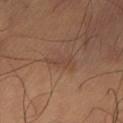Imaged during a routine full-body skin examination; the lesion was not biopsied and no histopathology is available. The total-body-photography lesion software estimated border irregularity of about 6.5 on a 0–10 scale, internal color variation of about 0 on a 0–10 scale, and peripheral color asymmetry of about 0. And it measured a nevus-likeness score of about 0/100 and a lesion-detection confidence of about 100/100. The recorded lesion diameter is about 3.5 mm. The lesion is on the lower back. A roughly 15 mm field-of-view crop from a total-body skin photograph. Captured under cross-polarized illumination. A male patient, aged around 60.